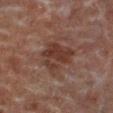{"biopsy_status": "not biopsied; imaged during a skin examination", "patient": {"sex": "male", "age_approx": 65}, "image": {"source": "total-body photography crop", "field_of_view_mm": 15}, "lesion_size": {"long_diameter_mm_approx": 4.5}, "automated_metrics": {"border_irregularity_0_10": 4.0, "color_variation_0_10": 3.5, "nevus_likeness_0_100": 5, "lesion_detection_confidence_0_100": 100}, "site": "leg", "lighting": "cross-polarized"}